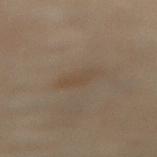  biopsy_status: not biopsied; imaged during a skin examination
  site: abdomen
  image:
    source: total-body photography crop
    field_of_view_mm: 15
  lesion_size:
    long_diameter_mm_approx: 3.5
  automated_metrics:
    area_mm2_approx: 6.0
    eccentricity: 0.8
    shape_asymmetry: 0.15
    border_irregularity_0_10: 2.5
    color_variation_0_10: 2.0
    peripheral_color_asymmetry: 0.5
    nevus_likeness_0_100: 10
    lesion_detection_confidence_0_100: 100
  patient:
    sex: female
    age_approx: 60
  lighting: cross-polarized